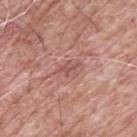* follow-up — imaged on a skin check; not biopsied
* image source — total-body-photography crop, ~15 mm field of view
* subject — male, approximately 60 years of age
* automated lesion analysis — a footprint of about 3.5 mm², a shape eccentricity near 0.8, and a shape-asymmetry score of about 0.3 (0 = symmetric); a border-irregularity index near 4/10; a nevus-likeness score of about 0/100 and lesion-presence confidence of about 95/100
* lighting — white-light illumination
* location — the upper back
* lesion size — about 3 mm